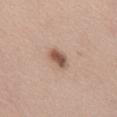{
  "biopsy_status": "not biopsied; imaged during a skin examination",
  "lesion_size": {
    "long_diameter_mm_approx": 3.5
  },
  "image": {
    "source": "total-body photography crop",
    "field_of_view_mm": 15
  },
  "patient": {
    "sex": "female",
    "age_approx": 50
  },
  "site": "mid back",
  "automated_metrics": {
    "area_mm2_approx": 4.5,
    "eccentricity": 0.85,
    "shape_asymmetry": 0.2,
    "nevus_likeness_0_100": 95,
    "lesion_detection_confidence_0_100": 100
  },
  "lighting": "white-light"
}